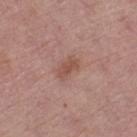Part of a total-body skin-imaging series; this lesion was reviewed on a skin check and was not flagged for biopsy. The patient is a female roughly 65 years of age. A lesion tile, about 15 mm wide, cut from a 3D total-body photograph. An algorithmic analysis of the crop reported a lesion area of about 4 mm², a shape eccentricity near 0.8, and a symmetry-axis asymmetry near 0.25. The software also gave a border-irregularity rating of about 3/10, a color-variation rating of about 1.5/10, and peripheral color asymmetry of about 0.5. Captured under white-light illumination. On the leg. The recorded lesion diameter is about 3 mm.